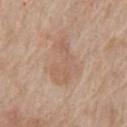<record>
  <biopsy_status>not biopsied; imaged during a skin examination</biopsy_status>
  <lesion_size>
    <long_diameter_mm_approx>6.0</long_diameter_mm_approx>
  </lesion_size>
  <patient>
    <sex>male</sex>
    <age_approx>65</age_approx>
  </patient>
  <lighting>white-light</lighting>
  <image>
    <source>total-body photography crop</source>
    <field_of_view_mm>15</field_of_view_mm>
  </image>
  <automated_metrics>
    <cielab_L>59</cielab_L>
    <cielab_a>18</cielab_a>
    <cielab_b>29</cielab_b>
    <vs_skin_contrast_norm>4.5</vs_skin_contrast_norm>
  </automated_metrics>
  <site>front of the torso</site>
</record>